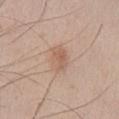<lesion>
<biopsy_status>not biopsied; imaged during a skin examination</biopsy_status>
<automated_metrics>
  <area_mm2_approx>7.0</area_mm2_approx>
  <eccentricity>0.8</eccentricity>
  <shape_asymmetry>0.2</shape_asymmetry>
  <border_irregularity_0_10>2.0</border_irregularity_0_10>
  <color_variation_0_10>3.5</color_variation_0_10>
  <peripheral_color_asymmetry>1.0</peripheral_color_asymmetry>
  <nevus_likeness_0_100>40</nevus_likeness_0_100>
  <lesion_detection_confidence_0_100>100</lesion_detection_confidence_0_100>
</automated_metrics>
<patient>
  <sex>male</sex>
  <age_approx>25</age_approx>
</patient>
<lesion_size>
  <long_diameter_mm_approx>4.0</long_diameter_mm_approx>
</lesion_size>
<site>left lower leg</site>
<image>
  <source>total-body photography crop</source>
  <field_of_view_mm>15</field_of_view_mm>
</image>
</lesion>